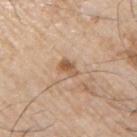No biopsy was performed on this lesion — it was imaged during a full skin examination and was not determined to be concerning.
The recorded lesion diameter is about 2.5 mm.
The subject is a male roughly 65 years of age.
Imaged with white-light lighting.
Located on the right upper arm.
The total-body-photography lesion software estimated an average lesion color of about L≈57 a*≈19 b*≈33 (CIELAB), a lesion–skin lightness drop of about 10, and a lesion-to-skin contrast of about 7.5 (normalized; higher = more distinct). It also reported a border-irregularity index near 3.5/10, internal color variation of about 4 on a 0–10 scale, and radial color variation of about 1.5. The software also gave a nevus-likeness score of about 80/100 and a lesion-detection confidence of about 100/100.
Cropped from a total-body skin-imaging series; the visible field is about 15 mm.